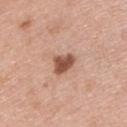{
  "biopsy_status": "not biopsied; imaged during a skin examination",
  "image": {
    "source": "total-body photography crop",
    "field_of_view_mm": 15
  },
  "site": "left upper arm",
  "lighting": "white-light",
  "automated_metrics": {
    "cielab_L": 53,
    "cielab_a": 23,
    "cielab_b": 29,
    "vs_skin_contrast_norm": 10.0,
    "border_irregularity_0_10": 3.0,
    "color_variation_0_10": 2.5,
    "nevus_likeness_0_100": 95,
    "lesion_detection_confidence_0_100": 100
  },
  "patient": {
    "sex": "male",
    "age_approx": 40
  }
}